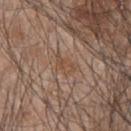The lesion was tiled from a total-body skin photograph and was not biopsied.
A male patient, about 45 years old.
This is a white-light tile.
Cropped from a total-body skin-imaging series; the visible field is about 15 mm.
Automated image analysis of the tile measured a mean CIELAB color near L≈49 a*≈17 b*≈29, about 6 CIELAB-L* units darker than the surrounding skin, and a normalized lesion–skin contrast near 5.5. And it measured a border-irregularity index near 4.5/10, a color-variation rating of about 2/10, and radial color variation of about 1. It also reported an automated nevus-likeness rating near 0 out of 100 and a detector confidence of about 80 out of 100 that the crop contains a lesion.
Approximately 3 mm at its widest.
From the upper back.